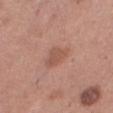Context: The recorded lesion diameter is about 3.5 mm. Imaged with white-light lighting. Automated image analysis of the tile measured a lesion color around L≈54 a*≈23 b*≈28 in CIELAB, roughly 8 lightness units darker than nearby skin, and a normalized border contrast of about 5.5. On the right thigh. A female subject about 40 years old. A region of skin cropped from a whole-body photographic capture, roughly 15 mm wide.